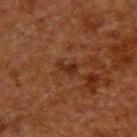  patient:
    sex: male
    age_approx: 60
  lesion_size:
    long_diameter_mm_approx: 2.5
  site: upper back
  image:
    source: total-body photography crop
    field_of_view_mm: 15
  lighting: cross-polarized